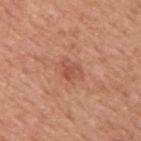Q: Was a biopsy performed?
A: no biopsy performed (imaged during a skin exam)
Q: How was the tile lit?
A: white-light illumination
Q: Lesion size?
A: about 3 mm
Q: Automated lesion metrics?
A: an average lesion color of about L≈53 a*≈26 b*≈31 (CIELAB) and a lesion-to-skin contrast of about 5.5 (normalized; higher = more distinct)
Q: Where on the body is the lesion?
A: the right upper arm
Q: How was this image acquired?
A: ~15 mm crop, total-body skin-cancer survey
Q: Patient demographics?
A: male, in their 50s